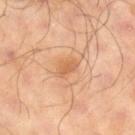anatomic site: the right lower leg | subject: male, aged 63–67 | TBP lesion metrics: an outline eccentricity of about 0.8 (0 = round, 1 = elongated); a border-irregularity rating of about 3.5/10 and peripheral color asymmetry of about 0.5 | lighting: cross-polarized illumination | acquisition: ~15 mm tile from a whole-body skin photo.